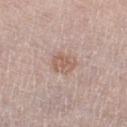The lesion was photographed on a routine skin check and not biopsied; there is no pathology result.
This is a white-light tile.
The lesion is on the left thigh.
Approximately 3 mm at its widest.
Cropped from a whole-body photographic skin survey; the tile spans about 15 mm.
A male subject, about 80 years old.
An algorithmic analysis of the crop reported a lesion color around L≈59 a*≈18 b*≈26 in CIELAB, a lesion–skin lightness drop of about 8, and a normalized border contrast of about 6.